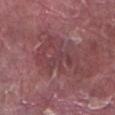<case>
<biopsy_status>not biopsied; imaged during a skin examination</biopsy_status>
<lesion_size>
  <long_diameter_mm_approx>7.5</long_diameter_mm_approx>
</lesion_size>
<site>right lower leg</site>
<image>
  <source>total-body photography crop</source>
  <field_of_view_mm>15</field_of_view_mm>
</image>
<patient>
  <sex>male</sex>
  <age_approx>40</age_approx>
</patient>
<lighting>white-light</lighting>
<automated_metrics>
  <cielab_L>41</cielab_L>
  <cielab_a>25</cielab_a>
  <cielab_b>17</cielab_b>
  <border_irregularity_0_10>9.0</border_irregularity_0_10>
  <color_variation_0_10>3.5</color_variation_0_10>
</automated_metrics>
</case>